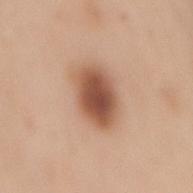<tbp_lesion>
<biopsy_status>not biopsied; imaged during a skin examination</biopsy_status>
<lesion_size>
  <long_diameter_mm_approx>5.5</long_diameter_mm_approx>
</lesion_size>
<automated_metrics>
  <eccentricity>0.8</eccentricity>
  <shape_asymmetry>0.15</shape_asymmetry>
  <cielab_L>54</cielab_L>
  <cielab_a>22</cielab_a>
  <cielab_b>31</cielab_b>
  <vs_skin_darker_L>16.0</vs_skin_darker_L>
  <vs_skin_contrast_norm>10.5</vs_skin_contrast_norm>
  <color_variation_0_10>6.0</color_variation_0_10>
  <peripheral_color_asymmetry>1.5</peripheral_color_asymmetry>
  <nevus_likeness_0_100>100</nevus_likeness_0_100>
  <lesion_detection_confidence_0_100>100</lesion_detection_confidence_0_100>
</automated_metrics>
<image>
  <source>total-body photography crop</source>
  <field_of_view_mm>15</field_of_view_mm>
</image>
<lighting>white-light</lighting>
<patient>
  <sex>female</sex>
  <age_approx>50</age_approx>
</patient>
<site>mid back</site>
</tbp_lesion>